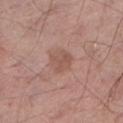follow-up: total-body-photography surveillance lesion; no biopsy
site: the left lower leg
image: ~15 mm crop, total-body skin-cancer survey
automated lesion analysis: a mean CIELAB color near L≈53 a*≈21 b*≈26, about 7 CIELAB-L* units darker than the surrounding skin, and a normalized lesion–skin contrast near 5.5; a border-irregularity rating of about 3.5/10 and peripheral color asymmetry of about 0.5
lesion size: ~2.5 mm (longest diameter)
lighting: white-light
patient: male, about 55 years old